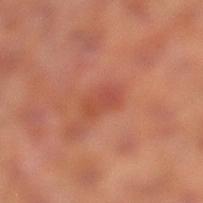- biopsy status: imaged on a skin check; not biopsied
- patient: male, in their mid-60s
- lesion diameter: ~3 mm (longest diameter)
- lighting: cross-polarized illumination
- acquisition: total-body-photography crop, ~15 mm field of view
- location: the left lower leg